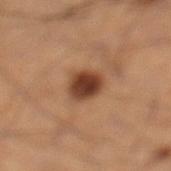This lesion was catalogued during total-body skin photography and was not selected for biopsy. A male patient, about 50 years old. A roughly 15 mm field-of-view crop from a total-body skin photograph. This is a cross-polarized tile. From the left leg. Measured at roughly 3.5 mm in maximum diameter.